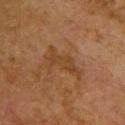{"biopsy_status": "not biopsied; imaged during a skin examination", "lesion_size": {"long_diameter_mm_approx": 5.0}, "image": {"source": "total-body photography crop", "field_of_view_mm": 15}, "patient": {"sex": "female", "age_approx": 55}, "site": "upper back", "lighting": "cross-polarized"}